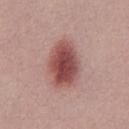Imaged during a routine full-body skin examination; the lesion was not biopsied and no histopathology is available. A 15 mm close-up extracted from a 3D total-body photography capture. The patient is a male roughly 30 years of age.